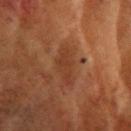{
  "biopsy_status": "not biopsied; imaged during a skin examination",
  "lesion_size": {
    "long_diameter_mm_approx": 4.5
  },
  "image": {
    "source": "total-body photography crop",
    "field_of_view_mm": 15
  },
  "automated_metrics": {
    "area_mm2_approx": 7.0,
    "eccentricity": 0.9,
    "shape_asymmetry": 0.3,
    "cielab_L": 28,
    "cielab_a": 18,
    "cielab_b": 25,
    "vs_skin_darker_L": 5.0,
    "vs_skin_contrast_norm": 5.0,
    "nevus_likeness_0_100": 0,
    "lesion_detection_confidence_0_100": 100
  },
  "patient": {
    "sex": "female",
    "age_approx": 80
  },
  "site": "head or neck",
  "lighting": "cross-polarized"
}